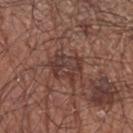Acquisition and patient details: Cropped from a total-body skin-imaging series; the visible field is about 15 mm. A male patient, aged approximately 65. The lesion is located on the left upper arm.